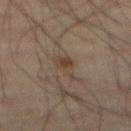biopsy_status: not biopsied; imaged during a skin examination
site: abdomen
patient:
  sex: male
  age_approx: 70
image:
  source: total-body photography crop
  field_of_view_mm: 15